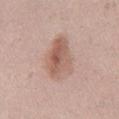Case summary:
- biopsy status · total-body-photography surveillance lesion; no biopsy
- lesion size · about 6 mm
- tile lighting · white-light
- patient · female, in their 20s
- body site · the right thigh
- image · ~15 mm tile from a whole-body skin photo